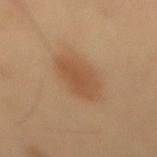Clinical impression:
No biopsy was performed on this lesion — it was imaged during a full skin examination and was not determined to be concerning.
Acquisition and patient details:
The lesion is located on the mid back. A region of skin cropped from a whole-body photographic capture, roughly 15 mm wide. The patient is a male roughly 55 years of age.